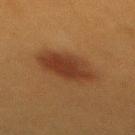Q: Was a biopsy performed?
A: catalogued during a skin exam; not biopsied
Q: What kind of image is this?
A: total-body-photography crop, ~15 mm field of view
Q: Patient demographics?
A: female, approximately 40 years of age
Q: What did automated image analysis measure?
A: a lesion area of about 17 mm², an outline eccentricity of about 0.8 (0 = round, 1 = elongated), and a shape-asymmetry score of about 0.15 (0 = symmetric); a mean CIELAB color near L≈31 a*≈19 b*≈28, about 8 CIELAB-L* units darker than the surrounding skin, and a normalized lesion–skin contrast near 8.5
Q: Lesion location?
A: the back
Q: Lesion size?
A: about 6 mm
Q: What lighting was used for the tile?
A: cross-polarized illumination